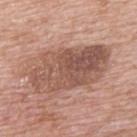Q: Was a biopsy performed?
A: total-body-photography surveillance lesion; no biopsy
Q: How large is the lesion?
A: about 9.5 mm
Q: What are the patient's age and sex?
A: male, aged approximately 70
Q: Lesion location?
A: the upper back
Q: What lighting was used for the tile?
A: white-light illumination
Q: How was this image acquired?
A: total-body-photography crop, ~15 mm field of view